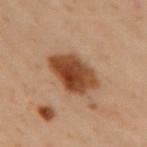notes — imaged on a skin check; not biopsied | size — ~6 mm (longest diameter) | tile lighting — cross-polarized illumination | image — total-body-photography crop, ~15 mm field of view | subject — female, roughly 60 years of age | location — the upper back | image-analysis metrics — a lesion area of about 16 mm², a shape eccentricity near 0.8, and two-axis asymmetry of about 0.15; a lesion-to-skin contrast of about 12 (normalized; higher = more distinct); a border-irregularity rating of about 2/10, internal color variation of about 5.5 on a 0–10 scale, and radial color variation of about 2; a nevus-likeness score of about 100/100 and a lesion-detection confidence of about 100/100.